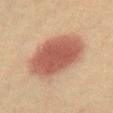Assessment:
No biopsy was performed on this lesion — it was imaged during a full skin examination and was not determined to be concerning.
Acquisition and patient details:
Captured under cross-polarized illumination. The lesion is located on the abdomen. The patient is a female aged 38 to 42. This image is a 15 mm lesion crop taken from a total-body photograph. Approximately 8 mm at its widest.